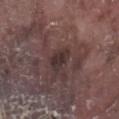follow-up — imaged on a skin check; not biopsied | subject — male, roughly 75 years of age | imaging modality — 15 mm crop, total-body photography | lesion size — about 3 mm | tile lighting — white-light | site — the right lower leg.